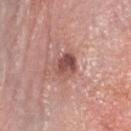workup = no biopsy performed (imaged during a skin exam) | subject = male, aged approximately 60 | imaging modality = ~15 mm tile from a whole-body skin photo | diameter = ~3 mm (longest diameter) | site = the head or neck.